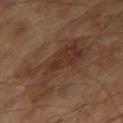Q: Was a biopsy performed?
A: imaged on a skin check; not biopsied
Q: Who is the patient?
A: male, roughly 65 years of age
Q: Illumination type?
A: cross-polarized illumination
Q: How was this image acquired?
A: ~15 mm crop, total-body skin-cancer survey
Q: What is the lesion's diameter?
A: about 9 mm